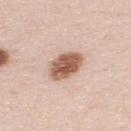Imaged during a routine full-body skin examination; the lesion was not biopsied and no histopathology is available. The lesion is on the upper back. A 15 mm crop from a total-body photograph taken for skin-cancer surveillance. The patient is a male aged around 35. The tile uses white-light illumination.